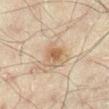Q: Was a biopsy performed?
A: catalogued during a skin exam; not biopsied
Q: What is the anatomic site?
A: the right lower leg
Q: What is the lesion's diameter?
A: about 2.5 mm
Q: What kind of image is this?
A: total-body-photography crop, ~15 mm field of view
Q: Patient demographics?
A: male, aged 43 to 47
Q: Automated lesion metrics?
A: an average lesion color of about L≈50 a*≈15 b*≈30 (CIELAB) and roughly 9 lightness units darker than nearby skin; a border-irregularity index near 2/10 and internal color variation of about 1.5 on a 0–10 scale; a classifier nevus-likeness of about 40/100 and lesion-presence confidence of about 100/100